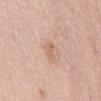Imaged during a routine full-body skin examination; the lesion was not biopsied and no histopathology is available. This is a white-light tile. A female subject, in their mid-60s. A region of skin cropped from a whole-body photographic capture, roughly 15 mm wide. From the mid back.